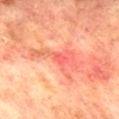Q: Is there a histopathology result?
A: catalogued during a skin exam; not biopsied
Q: Who is the patient?
A: male, in their mid- to late 70s
Q: Where on the body is the lesion?
A: the mid back
Q: What kind of image is this?
A: ~15 mm crop, total-body skin-cancer survey
Q: Illumination type?
A: cross-polarized illumination
Q: What is the lesion's diameter?
A: ~6.5 mm (longest diameter)
Q: Automated lesion metrics?
A: a mean CIELAB color near L≈55 a*≈33 b*≈31, a lesion–skin lightness drop of about 8, and a normalized lesion–skin contrast near 5.5